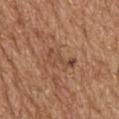{
  "biopsy_status": "not biopsied; imaged during a skin examination",
  "patient": {
    "sex": "male",
    "age_approx": 70
  },
  "lesion_size": {
    "long_diameter_mm_approx": 4.0
  },
  "site": "head or neck",
  "lighting": "white-light",
  "image": {
    "source": "total-body photography crop",
    "field_of_view_mm": 15
  }
}